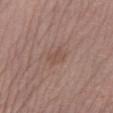Impression:
Recorded during total-body skin imaging; not selected for excision or biopsy.
Background:
A female subject, aged around 50. The lesion is located on the left lower leg. A region of skin cropped from a whole-body photographic capture, roughly 15 mm wide. Captured under white-light illumination.